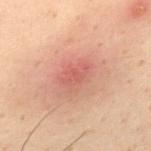Impression:
This lesion was catalogued during total-body skin photography and was not selected for biopsy.
Background:
Imaged with cross-polarized lighting. The total-body-photography lesion software estimated a footprint of about 4 mm² and a shape eccentricity near 0.8. The software also gave a lesion color around L≈47 a*≈25 b*≈24 in CIELAB, about 6 CIELAB-L* units darker than the surrounding skin, and a normalized lesion–skin contrast near 5. Located on the back. Cropped from a whole-body photographic skin survey; the tile spans about 15 mm. Longest diameter approximately 3 mm. A male subject aged 28–32.